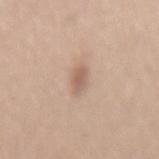Q: Is there a histopathology result?
A: no biopsy performed (imaged during a skin exam)
Q: How was this image acquired?
A: total-body-photography crop, ~15 mm field of view
Q: Where on the body is the lesion?
A: the mid back
Q: Who is the patient?
A: female, roughly 35 years of age
Q: How was the tile lit?
A: white-light